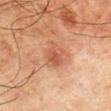Q: Is there a histopathology result?
A: imaged on a skin check; not biopsied
Q: What is the imaging modality?
A: total-body-photography crop, ~15 mm field of view
Q: Patient demographics?
A: male, aged approximately 80
Q: Lesion location?
A: the left thigh
Q: What lighting was used for the tile?
A: cross-polarized
Q: How large is the lesion?
A: ~4 mm (longest diameter)
Q: What did automated image analysis measure?
A: a footprint of about 9.5 mm², a shape eccentricity near 0.7, and a symmetry-axis asymmetry near 0.3; about 7 CIELAB-L* units darker than the surrounding skin and a lesion-to-skin contrast of about 6 (normalized; higher = more distinct); an automated nevus-likeness rating near 0 out of 100